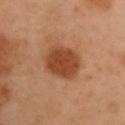workup: catalogued during a skin exam; not biopsied
lighting: cross-polarized
site: the right upper arm
subject: male, aged 53–57
diameter: about 4.5 mm
image-analysis metrics: a footprint of about 14 mm², a shape eccentricity near 0.5, and a symmetry-axis asymmetry near 0.1; an average lesion color of about L≈36 a*≈21 b*≈30 (CIELAB), about 10 CIELAB-L* units darker than the surrounding skin, and a normalized lesion–skin contrast near 9.5; a border-irregularity index near 1/10, a within-lesion color-variation index near 2/10, and a peripheral color-asymmetry measure near 0.5
acquisition: total-body-photography crop, ~15 mm field of view